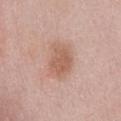workup = no biopsy performed (imaged during a skin exam) | subject = female, about 50 years old | size = ≈4.5 mm | lighting = white-light | site = the chest | imaging modality = 15 mm crop, total-body photography | automated metrics = a lesion area of about 11 mm² and a shape eccentricity near 0.75; a mean CIELAB color near L≈59 a*≈21 b*≈28 and a normalized border contrast of about 6.5; a classifier nevus-likeness of about 15/100 and a lesion-detection confidence of about 100/100.